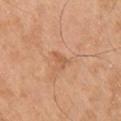workup = total-body-photography surveillance lesion; no biopsy | diameter = ~2.5 mm (longest diameter) | subject = male, approximately 60 years of age | lighting = cross-polarized | site = the leg | image = ~15 mm tile from a whole-body skin photo | automated lesion analysis = a lesion area of about 3 mm² and a shape eccentricity near 0.8; a mean CIELAB color near L≈51 a*≈21 b*≈32 and a lesion–skin lightness drop of about 6; a nevus-likeness score of about 0/100 and a detector confidence of about 100 out of 100 that the crop contains a lesion.